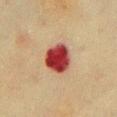notes — catalogued during a skin exam; not biopsied